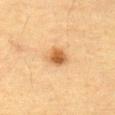Impression:
Imaged during a routine full-body skin examination; the lesion was not biopsied and no histopathology is available.
Context:
The lesion-visualizer software estimated about 13 CIELAB-L* units darker than the surrounding skin and a normalized border contrast of about 9. The analysis additionally found a border-irregularity rating of about 1.5/10, internal color variation of about 3.5 on a 0–10 scale, and radial color variation of about 1. A male patient, aged around 85 or older. About 2.5 mm across. A region of skin cropped from a whole-body photographic capture, roughly 15 mm wide. Located on the front of the torso. The tile uses cross-polarized illumination.